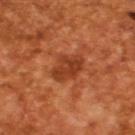{"image": {"source": "total-body photography crop", "field_of_view_mm": 15}, "lighting": "cross-polarized", "automated_metrics": {"area_mm2_approx": 8.0, "eccentricity": 0.7, "shape_asymmetry": 0.25, "cielab_L": 39, "cielab_a": 31, "cielab_b": 37, "vs_skin_contrast_norm": 7.5, "border_irregularity_0_10": 3.0, "color_variation_0_10": 3.5, "peripheral_color_asymmetry": 1.5, "nevus_likeness_0_100": 45, "lesion_detection_confidence_0_100": 100}, "lesion_size": {"long_diameter_mm_approx": 4.0}, "patient": {"sex": "male", "age_approx": 65}}